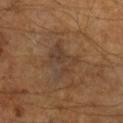workup: no biopsy performed (imaged during a skin exam) | automated metrics: an area of roughly 10 mm², an outline eccentricity of about 0.65 (0 = round, 1 = elongated), and a shape-asymmetry score of about 0.45 (0 = symmetric); a mean CIELAB color near L≈37 a*≈15 b*≈27, a lesion–skin lightness drop of about 6, and a normalized border contrast of about 6 | acquisition: total-body-photography crop, ~15 mm field of view | patient: male, aged around 65.